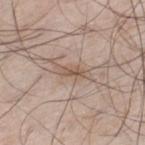biopsy_status: not biopsied; imaged during a skin examination